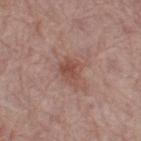Imaged during a routine full-body skin examination; the lesion was not biopsied and no histopathology is available.
Measured at roughly 2.5 mm in maximum diameter.
On the left thigh.
The subject is a female about 65 years old.
The tile uses white-light illumination.
The lesion-visualizer software estimated a mean CIELAB color near L≈49 a*≈22 b*≈26 and a normalized lesion–skin contrast near 6.5. And it measured border irregularity of about 2.5 on a 0–10 scale and peripheral color asymmetry of about 1.
A lesion tile, about 15 mm wide, cut from a 3D total-body photograph.